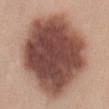Clinical impression:
This lesion was catalogued during total-body skin photography and was not selected for biopsy.
Context:
The lesion is on the back. A close-up tile cropped from a whole-body skin photograph, about 15 mm across. The subject is a female approximately 45 years of age.